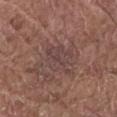Clinical impression: Captured during whole-body skin photography for melanoma surveillance; the lesion was not biopsied. Background: The lesion is on the front of the torso. A roughly 15 mm field-of-view crop from a total-body skin photograph. Imaged with white-light lighting. The lesion's longest dimension is about 5 mm. A male subject roughly 80 years of age.